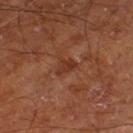This lesion was catalogued during total-body skin photography and was not selected for biopsy.
The total-body-photography lesion software estimated a footprint of about 4 mm². The software also gave a within-lesion color-variation index near 2.5/10 and peripheral color asymmetry of about 1. And it measured a classifier nevus-likeness of about 0/100 and lesion-presence confidence of about 100/100.
On the left thigh.
Imaged with cross-polarized lighting.
The subject is a male approximately 65 years of age.
Measured at roughly 2.5 mm in maximum diameter.
A 15 mm crop from a total-body photograph taken for skin-cancer surveillance.